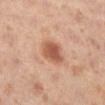Recorded during total-body skin imaging; not selected for excision or biopsy.
The lesion's longest dimension is about 3.5 mm.
Captured under cross-polarized illumination.
The subject is a female roughly 40 years of age.
From the right lower leg.
A roughly 15 mm field-of-view crop from a total-body skin photograph.